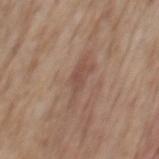{
  "biopsy_status": "not biopsied; imaged during a skin examination",
  "lesion_size": {
    "long_diameter_mm_approx": 6.0
  },
  "automated_metrics": {
    "nevus_likeness_0_100": 0,
    "lesion_detection_confidence_0_100": 55
  },
  "site": "mid back",
  "image": {
    "source": "total-body photography crop",
    "field_of_view_mm": 15
  },
  "lighting": "white-light",
  "patient": {
    "sex": "male",
    "age_approx": 70
  }
}